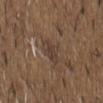Q: Is there a histopathology result?
A: total-body-photography surveillance lesion; no biopsy
Q: What is the imaging modality?
A: ~15 mm tile from a whole-body skin photo
Q: What is the anatomic site?
A: the front of the torso
Q: Lesion size?
A: ~3 mm (longest diameter)
Q: What lighting was used for the tile?
A: white-light illumination
Q: What are the patient's age and sex?
A: male, aged approximately 50